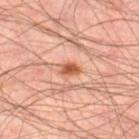No biopsy was performed on this lesion — it was imaged during a full skin examination and was not determined to be concerning. Imaged with cross-polarized lighting. Longest diameter approximately 2.5 mm. The subject is a male roughly 45 years of age. The lesion is on the right thigh. A 15 mm close-up extracted from a 3D total-body photography capture.